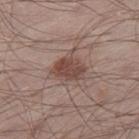Imaged during a routine full-body skin examination; the lesion was not biopsied and no histopathology is available. The lesion-visualizer software estimated a lesion area of about 8 mm² and an outline eccentricity of about 0.7 (0 = round, 1 = elongated). The lesion is located on the right thigh. Captured under white-light illumination. A male patient aged around 55. A 15 mm close-up extracted from a 3D total-body photography capture.